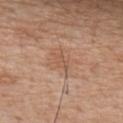Clinical impression:
Imaged during a routine full-body skin examination; the lesion was not biopsied and no histopathology is available.
Background:
A lesion tile, about 15 mm wide, cut from a 3D total-body photograph. This is a white-light tile. Automated tile analysis of the lesion measured a lesion area of about 3.5 mm², an outline eccentricity of about 0.8 (0 = round, 1 = elongated), and a shape-asymmetry score of about 0.3 (0 = symmetric). The software also gave border irregularity of about 3 on a 0–10 scale and internal color variation of about 1.5 on a 0–10 scale. The analysis additionally found an automated nevus-likeness rating near 0 out of 100 and a detector confidence of about 100 out of 100 that the crop contains a lesion. The lesion is on the upper back. Measured at roughly 2.5 mm in maximum diameter. The patient is a male roughly 65 years of age.